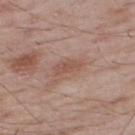* biopsy status · no biopsy performed (imaged during a skin exam)
* tile lighting · white-light
* lesion size · about 3 mm
* automated lesion analysis · a border-irregularity index near 3.5/10 and internal color variation of about 2 on a 0–10 scale
* body site · the upper back
* patient · male, in their mid- to late 60s
* acquisition · total-body-photography crop, ~15 mm field of view